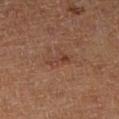Clinical impression:
Imaged during a routine full-body skin examination; the lesion was not biopsied and no histopathology is available.
Clinical summary:
This is a cross-polarized tile. A 15 mm close-up tile from a total-body photography series done for melanoma screening. The patient is female. The total-body-photography lesion software estimated an average lesion color of about L≈42 a*≈23 b*≈28 (CIELAB), a lesion–skin lightness drop of about 7, and a normalized border contrast of about 5.5. And it measured a border-irregularity index near 4.5/10, a color-variation rating of about 2.5/10, and peripheral color asymmetry of about 0.5. Approximately 3 mm at its widest. From the right lower leg.